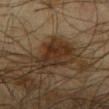- follow-up — total-body-photography surveillance lesion; no biopsy
- subject — male, about 65 years old
- automated metrics — a mean CIELAB color near L≈28 a*≈15 b*≈26, a lesion–skin lightness drop of about 9, and a normalized lesion–skin contrast near 9.5; a classifier nevus-likeness of about 40/100
- lighting — cross-polarized
- location — the upper back
- imaging modality — total-body-photography crop, ~15 mm field of view